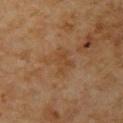Impression:
Captured during whole-body skin photography for melanoma surveillance; the lesion was not biopsied.
Background:
A male subject, aged approximately 60. A roughly 15 mm field-of-view crop from a total-body skin photograph. The lesion is on the left upper arm.